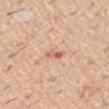Imaged with white-light lighting. From the right upper arm. A male subject in their 60s. The recorded lesion diameter is about 2.5 mm. A 15 mm close-up tile from a total-body photography series done for melanoma screening.